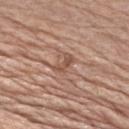Assessment: The lesion was photographed on a routine skin check and not biopsied; there is no pathology result. Background: The lesion is located on the front of the torso. A lesion tile, about 15 mm wide, cut from a 3D total-body photograph. A male patient, approximately 80 years of age.